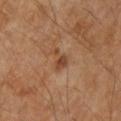The lesion was photographed on a routine skin check and not biopsied; there is no pathology result.
Imaged with cross-polarized lighting.
About 3 mm across.
A male patient, in their mid- to late 50s.
Located on the left upper arm.
A 15 mm close-up tile from a total-body photography series done for melanoma screening.